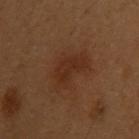Assessment: Imaged during a routine full-body skin examination; the lesion was not biopsied and no histopathology is available. Clinical summary: This image is a 15 mm lesion crop taken from a total-body photograph. The subject is a female aged 48 to 52. Located on the left upper arm. The lesion's longest dimension is about 5 mm. Automated image analysis of the tile measured a border-irregularity rating of about 4.5/10, internal color variation of about 2 on a 0–10 scale, and peripheral color asymmetry of about 0.5.